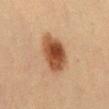Assessment:
The lesion was photographed on a routine skin check and not biopsied; there is no pathology result.
Background:
On the front of the torso. Captured under cross-polarized illumination. A close-up tile cropped from a whole-body skin photograph, about 15 mm across. The lesion's longest dimension is about 5.5 mm. The total-body-photography lesion software estimated an area of roughly 14 mm² and a symmetry-axis asymmetry near 0.2. The analysis additionally found a mean CIELAB color near L≈46 a*≈22 b*≈33, a lesion–skin lightness drop of about 15, and a normalized lesion–skin contrast near 11. The software also gave border irregularity of about 2 on a 0–10 scale and a peripheral color-asymmetry measure near 1.5. It also reported an automated nevus-likeness rating near 100 out of 100. The subject is a male in their mid- to late 30s.